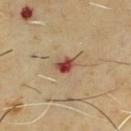– biopsy status: no biopsy performed (imaged during a skin exam)
– body site: the chest
– size: ~2.5 mm (longest diameter)
– acquisition: total-body-photography crop, ~15 mm field of view
– TBP lesion metrics: an automated nevus-likeness rating near 0 out of 100 and lesion-presence confidence of about 100/100
– tile lighting: cross-polarized
– patient: male, aged 63 to 67